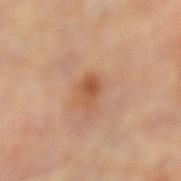| field | value |
|---|---|
| notes | no biopsy performed (imaged during a skin exam) |
| site | the left lower leg |
| diameter | about 3 mm |
| acquisition | ~15 mm tile from a whole-body skin photo |
| subject | female, roughly 60 years of age |
| lighting | cross-polarized illumination |